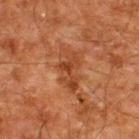Q: Was a biopsy performed?
A: catalogued during a skin exam; not biopsied
Q: What kind of image is this?
A: ~15 mm tile from a whole-body skin photo
Q: What lighting was used for the tile?
A: cross-polarized illumination
Q: Lesion size?
A: about 5 mm
Q: What are the patient's age and sex?
A: male, about 60 years old
Q: Automated lesion metrics?
A: about 7 CIELAB-L* units darker than the surrounding skin and a normalized lesion–skin contrast near 6.5
Q: What is the anatomic site?
A: the upper back